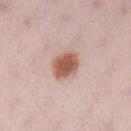Captured during whole-body skin photography for melanoma surveillance; the lesion was not biopsied.
Located on the leg.
This is a white-light tile.
About 3.5 mm across.
A region of skin cropped from a whole-body photographic capture, roughly 15 mm wide.
A female subject, aged 28 to 32.